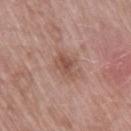notes: total-body-photography surveillance lesion; no biopsy
image source: ~15 mm tile from a whole-body skin photo
anatomic site: the right thigh
patient: female, aged 73 to 77